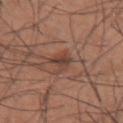No biopsy was performed on this lesion — it was imaged during a full skin examination and was not determined to be concerning. Located on the left upper arm. Approximately 3 mm at its widest. This image is a 15 mm lesion crop taken from a total-body photograph. A male patient, in their mid-30s. An algorithmic analysis of the crop reported a detector confidence of about 75 out of 100 that the crop contains a lesion.